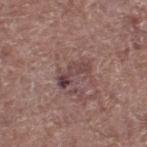The lesion was tiled from a total-body skin photograph and was not biopsied.
A lesion tile, about 15 mm wide, cut from a 3D total-body photograph.
An algorithmic analysis of the crop reported a mean CIELAB color near L≈42 a*≈20 b*≈18 and about 9 CIELAB-L* units darker than the surrounding skin. And it measured border irregularity of about 7 on a 0–10 scale and radial color variation of about 1.5. And it measured an automated nevus-likeness rating near 0 out of 100 and lesion-presence confidence of about 100/100.
The patient is a male aged 68 to 72.
Captured under white-light illumination.
Measured at roughly 4 mm in maximum diameter.
The lesion is located on the right lower leg.